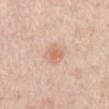Recorded during total-body skin imaging; not selected for excision or biopsy.
A region of skin cropped from a whole-body photographic capture, roughly 15 mm wide.
Imaged with white-light lighting.
Automated tile analysis of the lesion measured a lesion area of about 5 mm² and a symmetry-axis asymmetry near 0.2. It also reported a lesion color around L≈66 a*≈22 b*≈31 in CIELAB, roughly 9 lightness units darker than nearby skin, and a normalized border contrast of about 6. The analysis additionally found an automated nevus-likeness rating near 70 out of 100.
The lesion is located on the abdomen.
The recorded lesion diameter is about 2.5 mm.
A male subject, in their 50s.